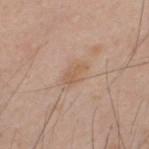Part of a total-body skin-imaging series; this lesion was reviewed on a skin check and was not flagged for biopsy. The tile uses white-light illumination. Automated image analysis of the tile measured a border-irregularity rating of about 4/10 and a color-variation rating of about 2/10. A 15 mm close-up extracted from a 3D total-body photography capture. Located on the back. The lesion's longest dimension is about 3.5 mm. A male subject, about 35 years old.